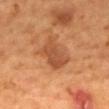notes: catalogued during a skin exam; not biopsied
TBP lesion metrics: a lesion color around L≈47 a*≈25 b*≈35 in CIELAB, roughly 9 lightness units darker than nearby skin, and a lesion-to-skin contrast of about 7 (normalized; higher = more distinct); a nevus-likeness score of about 25/100 and lesion-presence confidence of about 100/100
diameter: about 4.5 mm
location: the mid back
subject: male, approximately 55 years of age
lighting: cross-polarized illumination
image: ~15 mm crop, total-body skin-cancer survey